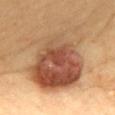Impression: Recorded during total-body skin imaging; not selected for excision or biopsy. Acquisition and patient details: A 15 mm close-up tile from a total-body photography series done for melanoma screening. From the mid back. An algorithmic analysis of the crop reported an average lesion color of about L≈55 a*≈22 b*≈35 (CIELAB), a lesion–skin lightness drop of about 15, and a normalized border contrast of about 9.5. Imaged with cross-polarized lighting. A female patient about 60 years old.